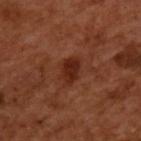Cropped from a whole-body photographic skin survey; the tile spans about 15 mm.
A male subject aged around 50.
From the upper back.
Imaged with cross-polarized lighting.
The recorded lesion diameter is about 3 mm.
An algorithmic analysis of the crop reported a color-variation rating of about 2/10 and radial color variation of about 0.5. The analysis additionally found a nevus-likeness score of about 80/100 and a lesion-detection confidence of about 100/100.